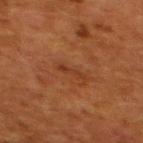biopsy status — imaged on a skin check; not biopsied | patient — female, aged around 50 | site — the upper back | TBP lesion metrics — a lesion color around L≈30 a*≈22 b*≈29 in CIELAB, about 5 CIELAB-L* units darker than the surrounding skin, and a normalized lesion–skin contrast near 5.5; border irregularity of about 5.5 on a 0–10 scale and internal color variation of about 0 on a 0–10 scale; a classifier nevus-likeness of about 0/100 and a lesion-detection confidence of about 100/100 | lighting — cross-polarized | image source — total-body-photography crop, ~15 mm field of view | size — ~3 mm (longest diameter).